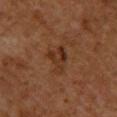Impression: Recorded during total-body skin imaging; not selected for excision or biopsy.